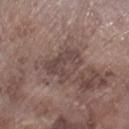| key | value |
|---|---|
| biopsy status | imaged on a skin check; not biopsied |
| subject | male, roughly 75 years of age |
| lighting | white-light illumination |
| body site | the right lower leg |
| image source | ~15 mm tile from a whole-body skin photo |
| size | ~4.5 mm (longest diameter) |